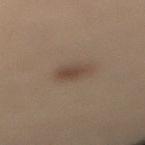Recorded during total-body skin imaging; not selected for excision or biopsy.
A 15 mm close-up tile from a total-body photography series done for melanoma screening.
About 2.5 mm across.
The subject is a female about 50 years old.
From the right lower leg.